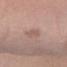biopsy_status: not biopsied; imaged during a skin examination